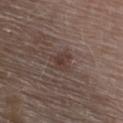{"biopsy_status": "not biopsied; imaged during a skin examination", "lighting": "white-light", "lesion_size": {"long_diameter_mm_approx": 2.5}, "patient": {"sex": "female", "age_approx": 65}, "automated_metrics": {"eccentricity": 0.5, "shape_asymmetry": 0.35, "cielab_L": 37, "cielab_a": 16, "cielab_b": 20, "vs_skin_darker_L": 6.0, "nevus_likeness_0_100": 0}, "site": "upper back", "image": {"source": "total-body photography crop", "field_of_view_mm": 15}}